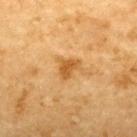Q: Was this lesion biopsied?
A: total-body-photography surveillance lesion; no biopsy
Q: What lighting was used for the tile?
A: cross-polarized
Q: What is the lesion's diameter?
A: ~2.5 mm (longest diameter)
Q: Patient demographics?
A: male, roughly 85 years of age or older
Q: What kind of image is this?
A: total-body-photography crop, ~15 mm field of view
Q: Lesion location?
A: the upper back